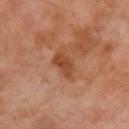No biopsy was performed on this lesion — it was imaged during a full skin examination and was not determined to be concerning. The patient is a male aged 68 to 72. On the left upper arm. Cropped from a total-body skin-imaging series; the visible field is about 15 mm. Captured under cross-polarized illumination. Measured at roughly 5 mm in maximum diameter. Automated image analysis of the tile measured a lesion area of about 6.5 mm², an outline eccentricity of about 0.95 (0 = round, 1 = elongated), and a shape-asymmetry score of about 0.3 (0 = symmetric). And it measured a lesion–skin lightness drop of about 9 and a normalized lesion–skin contrast near 7.5. It also reported a border-irregularity rating of about 4/10, a within-lesion color-variation index near 2.5/10, and a peripheral color-asymmetry measure near 1. And it measured a nevus-likeness score of about 0/100 and a lesion-detection confidence of about 100/100.